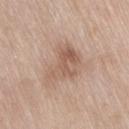Part of a total-body skin-imaging series; this lesion was reviewed on a skin check and was not flagged for biopsy.
Imaged with white-light lighting.
The lesion is located on the leg.
Automated tile analysis of the lesion measured a lesion–skin lightness drop of about 9 and a normalized lesion–skin contrast near 6. The software also gave a border-irregularity index near 4.5/10 and a color-variation rating of about 4.5/10. And it measured an automated nevus-likeness rating near 5 out of 100 and a lesion-detection confidence of about 100/100.
Cropped from a whole-body photographic skin survey; the tile spans about 15 mm.
The subject is a female roughly 75 years of age.
Measured at roughly 6 mm in maximum diameter.